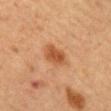Q: What is the imaging modality?
A: 15 mm crop, total-body photography
Q: Lesion size?
A: ≈3.5 mm
Q: How was the tile lit?
A: cross-polarized
Q: Who is the patient?
A: female, roughly 60 years of age
Q: Lesion location?
A: the mid back